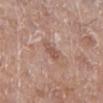Clinical impression: No biopsy was performed on this lesion — it was imaged during a full skin examination and was not determined to be concerning. Acquisition and patient details: Imaged with white-light lighting. From the leg. About 2.5 mm across. The patient is a female aged around 75. A lesion tile, about 15 mm wide, cut from a 3D total-body photograph.